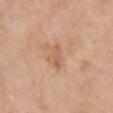Part of a total-body skin-imaging series; this lesion was reviewed on a skin check and was not flagged for biopsy. A 15 mm close-up tile from a total-body photography series done for melanoma screening. This is a white-light tile. Located on the chest. Longest diameter approximately 3.5 mm. A female subject, aged around 55.